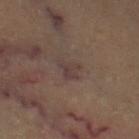Part of a total-body skin-imaging series; this lesion was reviewed on a skin check and was not flagged for biopsy.
The recorded lesion diameter is about 2.5 mm.
On the left thigh.
A subject in their 60s.
The tile uses cross-polarized illumination.
A roughly 15 mm field-of-view crop from a total-body skin photograph.